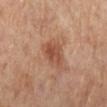The lesion was photographed on a routine skin check and not biopsied; there is no pathology result. Automated image analysis of the tile measured a border-irregularity rating of about 3.5/10, a within-lesion color-variation index near 4/10, and a peripheral color-asymmetry measure near 1.5. The software also gave a nevus-likeness score of about 55/100 and a detector confidence of about 100 out of 100 that the crop contains a lesion. The lesion is on the right lower leg. This image is a 15 mm lesion crop taken from a total-body photograph. A female subject, aged 53–57. This is a cross-polarized tile.